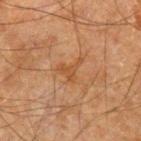Imaged during a routine full-body skin examination; the lesion was not biopsied and no histopathology is available.
Imaged with cross-polarized lighting.
A male patient, aged approximately 65.
The total-body-photography lesion software estimated an area of roughly 4 mm² and two-axis asymmetry of about 0.75. The analysis additionally found a border-irregularity index near 7.5/10, a color-variation rating of about 0.5/10, and a peripheral color-asymmetry measure near 0. And it measured a nevus-likeness score of about 0/100.
Measured at roughly 3 mm in maximum diameter.
A roughly 15 mm field-of-view crop from a total-body skin photograph.
The lesion is located on the left forearm.